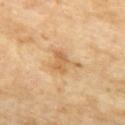Q: Was this lesion biopsied?
A: total-body-photography surveillance lesion; no biopsy
Q: What are the patient's age and sex?
A: female, approximately 75 years of age
Q: How large is the lesion?
A: about 3.5 mm
Q: Illumination type?
A: cross-polarized illumination
Q: What is the anatomic site?
A: the chest
Q: How was this image acquired?
A: ~15 mm crop, total-body skin-cancer survey